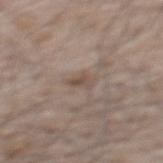Q: Is there a histopathology result?
A: catalogued during a skin exam; not biopsied
Q: How large is the lesion?
A: ~3 mm (longest diameter)
Q: Lesion location?
A: the upper back
Q: How was this image acquired?
A: total-body-photography crop, ~15 mm field of view
Q: Patient demographics?
A: male, about 65 years old
Q: Illumination type?
A: white-light